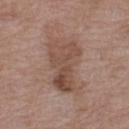A 15 mm crop from a total-body photograph taken for skin-cancer surveillance. Measured at roughly 6.5 mm in maximum diameter. From the leg. A female subject aged approximately 70. Automated image analysis of the tile measured two-axis asymmetry of about 0.4. It also reported a border-irregularity index near 4.5/10, a color-variation rating of about 5.5/10, and radial color variation of about 2.